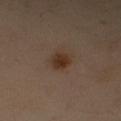Q: Was this lesion biopsied?
A: no biopsy performed (imaged during a skin exam)
Q: Patient demographics?
A: male, approximately 55 years of age
Q: What is the imaging modality?
A: ~15 mm crop, total-body skin-cancer survey
Q: What did automated image analysis measure?
A: a border-irregularity index near 1.5/10, internal color variation of about 2.5 on a 0–10 scale, and peripheral color asymmetry of about 0.5; a nevus-likeness score of about 100/100 and lesion-presence confidence of about 100/100
Q: What is the lesion's diameter?
A: ≈2.5 mm
Q: Where on the body is the lesion?
A: the right upper arm